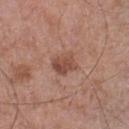Clinical impression:
This lesion was catalogued during total-body skin photography and was not selected for biopsy.
Clinical summary:
Cropped from a total-body skin-imaging series; the visible field is about 15 mm. Imaged with white-light lighting. On the left lower leg. A male subject, aged 58–62. Longest diameter approximately 3 mm.